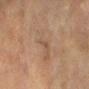Notes:
– follow-up · total-body-photography surveillance lesion; no biopsy
– subject · female, about 80 years old
– image source · total-body-photography crop, ~15 mm field of view
– body site · the right forearm